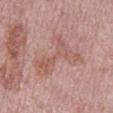Findings:
– biopsy status — imaged on a skin check; not biopsied
– lesion diameter — ~7 mm (longest diameter)
– subject — male, aged around 75
– image — 15 mm crop, total-body photography
– illumination — white-light illumination
– anatomic site — the mid back